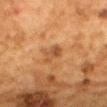Recorded during total-body skin imaging; not selected for excision or biopsy. Measured at roughly 3 mm in maximum diameter. Cropped from a whole-body photographic skin survey; the tile spans about 15 mm. An algorithmic analysis of the crop reported a footprint of about 3.5 mm², a shape eccentricity near 0.9, and a shape-asymmetry score of about 0.35 (0 = symmetric). It also reported about 8 CIELAB-L* units darker than the surrounding skin. And it measured an automated nevus-likeness rating near 0 out of 100. The lesion is on the mid back. Captured under cross-polarized illumination. The subject is a female aged 48 to 52.